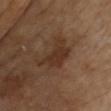No biopsy was performed on this lesion — it was imaged during a full skin examination and was not determined to be concerning. A lesion tile, about 15 mm wide, cut from a 3D total-body photograph. On the chest. A female patient aged around 65. The lesion's longest dimension is about 5 mm.